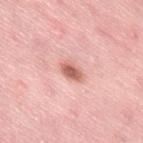{"site": "right thigh", "image": {"source": "total-body photography crop", "field_of_view_mm": 15}, "patient": {"sex": "female", "age_approx": 40}}